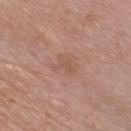workup: imaged on a skin check; not biopsied
automated metrics: an area of roughly 4.5 mm², an outline eccentricity of about 0.75 (0 = round, 1 = elongated), and a symmetry-axis asymmetry near 0.45; an automated nevus-likeness rating near 0 out of 100
site: the chest
image: ~15 mm tile from a whole-body skin photo
patient: male, in their 50s